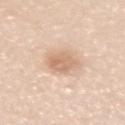Clinical impression:
No biopsy was performed on this lesion — it was imaged during a full skin examination and was not determined to be concerning.
Acquisition and patient details:
The lesion is on the right upper arm. A roughly 15 mm field-of-view crop from a total-body skin photograph. A female patient, aged 38–42. The tile uses white-light illumination. Measured at roughly 3.5 mm in maximum diameter.